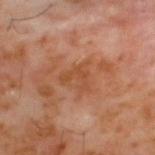Captured during whole-body skin photography for melanoma surveillance; the lesion was not biopsied. A lesion tile, about 15 mm wide, cut from a 3D total-body photograph. Captured under cross-polarized illumination. Approximately 3.5 mm at its widest. A male patient, about 60 years old. On the upper back.